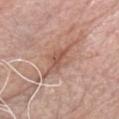Assessment: Part of a total-body skin-imaging series; this lesion was reviewed on a skin check and was not flagged for biopsy. Image and clinical context: Longest diameter approximately 7 mm. The tile uses white-light illumination. Located on the chest. A 15 mm crop from a total-body photograph taken for skin-cancer surveillance. A male patient about 65 years old.